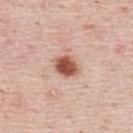<record>
<biopsy_status>not biopsied; imaged during a skin examination</biopsy_status>
<site>mid back</site>
<automated_metrics>
  <eccentricity>0.7</eccentricity>
  <shape_asymmetry>0.25</shape_asymmetry>
  <cielab_L>54</cielab_L>
  <cielab_a>24</cielab_a>
  <cielab_b>30</cielab_b>
  <vs_skin_darker_L>18.0</vs_skin_darker_L>
  <vs_skin_contrast_norm>11.5</vs_skin_contrast_norm>
  <border_irregularity_0_10>2.0</border_irregularity_0_10>
</automated_metrics>
<patient>
  <sex>male</sex>
  <age_approx>45</age_approx>
</patient>
<image>
  <source>total-body photography crop</source>
  <field_of_view_mm>15</field_of_view_mm>
</image>
<lesion_size>
  <long_diameter_mm_approx>3.5</long_diameter_mm_approx>
</lesion_size>
</record>